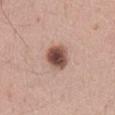biopsy status = no biopsy performed (imaged during a skin exam); patient = male, aged around 60; site = the abdomen; image source = ~15 mm crop, total-body skin-cancer survey.